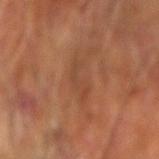Clinical impression:
The lesion was tiled from a total-body skin photograph and was not biopsied.
Context:
Imaged with cross-polarized lighting. A 15 mm close-up tile from a total-body photography series done for melanoma screening. The lesion is located on the left forearm. A male patient aged 63 to 67.